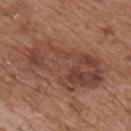follow-up = no biopsy performed (imaged during a skin exam) | subject = male, roughly 65 years of age | acquisition = ~15 mm crop, total-body skin-cancer survey | tile lighting = white-light illumination | automated lesion analysis = a footprint of about 35 mm² and an eccentricity of roughly 0.9; a lesion color around L≈43 a*≈22 b*≈26 in CIELAB, about 9 CIELAB-L* units darker than the surrounding skin, and a normalized lesion–skin contrast near 7 | size = ≈10 mm | location = the mid back.